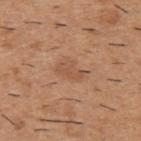Part of a total-body skin-imaging series; this lesion was reviewed on a skin check and was not flagged for biopsy.
A male patient, in their 40s.
A roughly 15 mm field-of-view crop from a total-body skin photograph.
This is a white-light tile.
The lesion is on the upper back.